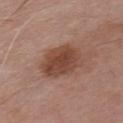Case summary:
• image-analysis metrics: a nevus-likeness score of about 90/100
• location: the chest
• size: ~5 mm (longest diameter)
• tile lighting: white-light illumination
• image source: total-body-photography crop, ~15 mm field of view
• subject: male, approximately 70 years of age